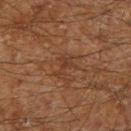Case summary:
– follow-up — total-body-photography surveillance lesion; no biopsy
– patient — male, approximately 60 years of age
– body site — the right lower leg
– image — total-body-photography crop, ~15 mm field of view
– size — about 3.5 mm
– illumination — cross-polarized illumination
– automated lesion analysis — a mean CIELAB color near L≈28 a*≈16 b*≈24, about 5 CIELAB-L* units darker than the surrounding skin, and a normalized lesion–skin contrast near 5; a border-irregularity index near 5.5/10, a color-variation rating of about 2/10, and peripheral color asymmetry of about 0.5; a nevus-likeness score of about 0/100 and a detector confidence of about 95 out of 100 that the crop contains a lesion